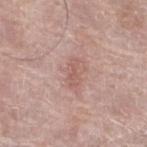<record>
  <site>left lower leg</site>
  <lighting>white-light</lighting>
  <image>
    <source>total-body photography crop</source>
    <field_of_view_mm>15</field_of_view_mm>
  </image>
  <lesion_size>
    <long_diameter_mm_approx>3.0</long_diameter_mm_approx>
  </lesion_size>
  <patient>
    <sex>female</sex>
    <age_approx>60</age_approx>
  </patient>
</record>